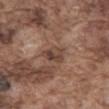Captured during whole-body skin photography for melanoma surveillance; the lesion was not biopsied.
The subject is a male aged around 75.
The lesion is located on the abdomen.
Measured at roughly 3.5 mm in maximum diameter.
Automated tile analysis of the lesion measured border irregularity of about 3 on a 0–10 scale, internal color variation of about 6.5 on a 0–10 scale, and peripheral color asymmetry of about 2.5.
The tile uses white-light illumination.
A lesion tile, about 15 mm wide, cut from a 3D total-body photograph.